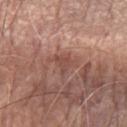Q: Was this lesion biopsied?
A: imaged on a skin check; not biopsied
Q: What lighting was used for the tile?
A: white-light illumination
Q: Who is the patient?
A: male, aged 68–72
Q: Where on the body is the lesion?
A: the left forearm
Q: What kind of image is this?
A: ~15 mm tile from a whole-body skin photo
Q: Lesion size?
A: ≈3 mm